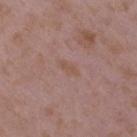Clinical impression:
Captured during whole-body skin photography for melanoma surveillance; the lesion was not biopsied.
Background:
The lesion's longest dimension is about 2.5 mm. The tile uses white-light illumination. A 15 mm close-up extracted from a 3D total-body photography capture. A female patient aged around 35. Located on the right upper arm. The lesion-visualizer software estimated an area of roughly 2.5 mm² and two-axis asymmetry of about 0.2. It also reported border irregularity of about 2.5 on a 0–10 scale and a peripheral color-asymmetry measure near 0.